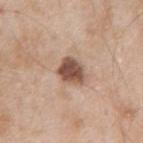The lesion's longest dimension is about 3.5 mm. A male subject, approximately 55 years of age. Imaged with white-light lighting. A 15 mm close-up extracted from a 3D total-body photography capture. Automated tile analysis of the lesion measured an area of roughly 7.5 mm² and an outline eccentricity of about 0.6 (0 = round, 1 = elongated). From the arm.